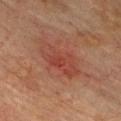follow-up: total-body-photography surveillance lesion; no biopsy | subject: male, in their mid- to late 70s | lighting: cross-polarized illumination | size: ≈6 mm | body site: the chest | image source: total-body-photography crop, ~15 mm field of view.